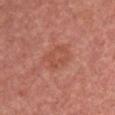No biopsy was performed on this lesion — it was imaged during a full skin examination and was not determined to be concerning.
A female patient approximately 60 years of age.
Located on the chest.
This is a white-light tile.
A close-up tile cropped from a whole-body skin photograph, about 15 mm across.